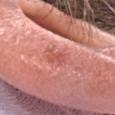<record>
  <biopsy_status>not biopsied; imaged during a skin examination</biopsy_status>
</record>